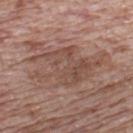Q: Is there a histopathology result?
A: imaged on a skin check; not biopsied
Q: Who is the patient?
A: male, aged around 70
Q: What is the imaging modality?
A: total-body-photography crop, ~15 mm field of view
Q: Where on the body is the lesion?
A: the back
Q: What lighting was used for the tile?
A: white-light illumination